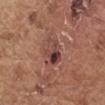Q: Is there a histopathology result?
A: imaged on a skin check; not biopsied
Q: Lesion location?
A: the left upper arm
Q: Lesion size?
A: ~3.5 mm (longest diameter)
Q: What are the patient's age and sex?
A: male, in their 70s
Q: What lighting was used for the tile?
A: white-light
Q: What did automated image analysis measure?
A: a normalized lesion–skin contrast near 8.5; a nevus-likeness score of about 20/100 and a lesion-detection confidence of about 100/100
Q: What is the imaging modality?
A: ~15 mm crop, total-body skin-cancer survey